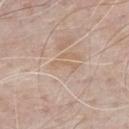The lesion was tiled from a total-body skin photograph and was not biopsied.
This is a white-light tile.
On the chest.
Cropped from a total-body skin-imaging series; the visible field is about 15 mm.
The recorded lesion diameter is about 3.5 mm.
An algorithmic analysis of the crop reported a footprint of about 3 mm², a shape eccentricity near 0.95, and a symmetry-axis asymmetry near 0.45. It also reported a mean CIELAB color near L≈63 a*≈15 b*≈30 and about 5 CIELAB-L* units darker than the surrounding skin. The software also gave a classifier nevus-likeness of about 0/100 and a detector confidence of about 55 out of 100 that the crop contains a lesion.
A male patient aged around 70.